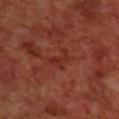This lesion was catalogued during total-body skin photography and was not selected for biopsy. Located on the upper back. Imaged with cross-polarized lighting. The lesion's longest dimension is about 3 mm. A male subject, aged around 70. Cropped from a total-body skin-imaging series; the visible field is about 15 mm. The lesion-visualizer software estimated an eccentricity of roughly 0.85 and a symmetry-axis asymmetry near 0.65.